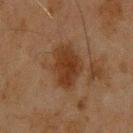Case summary:
* biopsy status: imaged on a skin check; not biopsied
* TBP lesion metrics: a lesion area of about 14 mm², a shape eccentricity near 0.7, and a symmetry-axis asymmetry near 0.2; a normalized lesion–skin contrast near 8.5; a classifier nevus-likeness of about 25/100
* image source: ~15 mm tile from a whole-body skin photo
* body site: the upper back
* patient: male, approximately 45 years of age